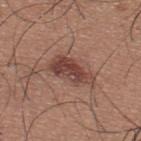Q: Was a biopsy performed?
A: total-body-photography surveillance lesion; no biopsy
Q: What is the imaging modality?
A: 15 mm crop, total-body photography
Q: What is the anatomic site?
A: the upper back
Q: Who is the patient?
A: male, aged approximately 35
Q: How large is the lesion?
A: about 5 mm
Q: What did automated image analysis measure?
A: a footprint of about 9 mm² and a symmetry-axis asymmetry near 0.3; an average lesion color of about L≈43 a*≈22 b*≈24 (CIELAB), roughly 12 lightness units darker than nearby skin, and a normalized border contrast of about 9.5; a lesion-detection confidence of about 100/100
Q: What lighting was used for the tile?
A: white-light illumination